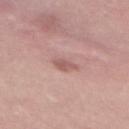Q: Is there a histopathology result?
A: no biopsy performed (imaged during a skin exam)
Q: Who is the patient?
A: female, aged 23–27
Q: Where on the body is the lesion?
A: the upper back
Q: Automated lesion metrics?
A: a mean CIELAB color near L≈57 a*≈22 b*≈22 and a normalized lesion–skin contrast near 6; border irregularity of about 3 on a 0–10 scale and a peripheral color-asymmetry measure near 0.5
Q: How was this image acquired?
A: ~15 mm tile from a whole-body skin photo
Q: What lighting was used for the tile?
A: white-light
Q: Lesion size?
A: ≈2.5 mm